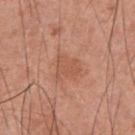Q: Was this lesion biopsied?
A: catalogued during a skin exam; not biopsied
Q: What is the imaging modality?
A: total-body-photography crop, ~15 mm field of view
Q: What are the patient's age and sex?
A: male, aged approximately 55
Q: What is the anatomic site?
A: the chest